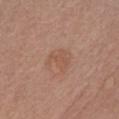workup = imaged on a skin check; not biopsied
lesion diameter = ≈3 mm
imaging modality = ~15 mm tile from a whole-body skin photo
subject = female, aged 48 to 52
automated metrics = a lesion color around L≈53 a*≈20 b*≈29 in CIELAB, roughly 6 lightness units darker than nearby skin, and a normalized lesion–skin contrast near 4.5; internal color variation of about 1.5 on a 0–10 scale and radial color variation of about 0.5; a nevus-likeness score of about 5/100 and a detector confidence of about 100 out of 100 that the crop contains a lesion
tile lighting = white-light illumination
anatomic site = the chest